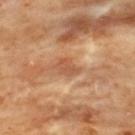* notes: imaged on a skin check; not biopsied
* lesion size: about 3 mm
* imaging modality: ~15 mm crop, total-body skin-cancer survey
* site: the back
* automated metrics: a mean CIELAB color near L≈56 a*≈25 b*≈37, about 9 CIELAB-L* units darker than the surrounding skin, and a normalized lesion–skin contrast near 6.5; a border-irregularity rating of about 2.5/10 and peripheral color asymmetry of about 0.5; a classifier nevus-likeness of about 0/100 and a detector confidence of about 100 out of 100 that the crop contains a lesion
* tile lighting: cross-polarized illumination